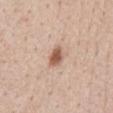Notes:
* workup: total-body-photography surveillance lesion; no biopsy
* image: ~15 mm crop, total-body skin-cancer survey
* patient: male, aged 58 to 62
* tile lighting: white-light illumination
* location: the abdomen
* image-analysis metrics: a footprint of about 4.5 mm², a shape eccentricity near 0.75, and a shape-asymmetry score of about 0.25 (0 = symmetric); border irregularity of about 2 on a 0–10 scale and a color-variation rating of about 3/10